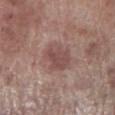Acquisition and patient details:
A roughly 15 mm field-of-view crop from a total-body skin photograph. This is a white-light tile. Automated image analysis of the tile measured about 9 CIELAB-L* units darker than the surrounding skin and a normalized lesion–skin contrast near 6.5. The patient is a male roughly 70 years of age. The lesion's longest dimension is about 4.5 mm. Located on the right lower leg.